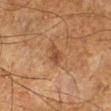Recorded during total-body skin imaging; not selected for excision or biopsy. On the right lower leg. A male patient, aged approximately 60. Cropped from a whole-body photographic skin survey; the tile spans about 15 mm.